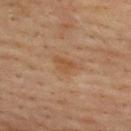Q: Is there a histopathology result?
A: catalogued during a skin exam; not biopsied
Q: Where on the body is the lesion?
A: the upper back
Q: What are the patient's age and sex?
A: male, about 40 years old
Q: What is the imaging modality?
A: total-body-photography crop, ~15 mm field of view